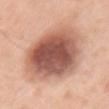follow-up: total-body-photography surveillance lesion; no biopsy
image source: 15 mm crop, total-body photography
anatomic site: the chest
image-analysis metrics: an eccentricity of roughly 0.75; a mean CIELAB color near L≈57 a*≈23 b*≈28; border irregularity of about 1.5 on a 0–10 scale, a color-variation rating of about 8/10, and a peripheral color-asymmetry measure near 2
patient: female, in their 50s